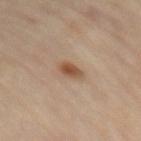Clinical impression: Recorded during total-body skin imaging; not selected for excision or biopsy. Clinical summary: A female patient, about 60 years old. Imaged with cross-polarized lighting. The lesion is located on the left thigh. A roughly 15 mm field-of-view crop from a total-body skin photograph. Automated tile analysis of the lesion measured a lesion color around L≈51 a*≈18 b*≈31 in CIELAB, roughly 11 lightness units darker than nearby skin, and a lesion-to-skin contrast of about 8.5 (normalized; higher = more distinct). The software also gave an automated nevus-likeness rating near 95 out of 100 and a lesion-detection confidence of about 100/100. About 2.5 mm across.